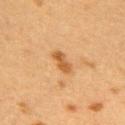site = the right upper arm
automated lesion analysis = a border-irregularity rating of about 2.5/10 and a within-lesion color-variation index near 2/10; a classifier nevus-likeness of about 80/100 and lesion-presence confidence of about 100/100
image source = total-body-photography crop, ~15 mm field of view
tile lighting = cross-polarized
patient = female, roughly 40 years of age
size = ≈3 mm